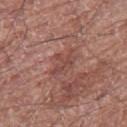<lesion>
  <biopsy_status>not biopsied; imaged during a skin examination</biopsy_status>
  <lesion_size>
    <long_diameter_mm_approx>4.0</long_diameter_mm_approx>
  </lesion_size>
  <image>
    <source>total-body photography crop</source>
    <field_of_view_mm>15</field_of_view_mm>
  </image>
  <site>right thigh</site>
  <patient>
    <sex>male</sex>
    <age_approx>75</age_approx>
  </patient>
  <lighting>white-light</lighting>
</lesion>